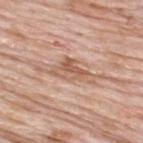A male patient in their 60s. The recorded lesion diameter is about 5 mm. The lesion is located on the back. Cropped from a whole-body photographic skin survey; the tile spans about 15 mm. Captured under white-light illumination.